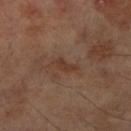{
  "biopsy_status": "not biopsied; imaged during a skin examination",
  "image": {
    "source": "total-body photography crop",
    "field_of_view_mm": 15
  },
  "patient": {
    "sex": "male",
    "age_approx": 70
  },
  "lesion_size": {
    "long_diameter_mm_approx": 2.5
  },
  "site": "right lower leg"
}